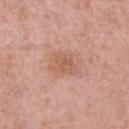  biopsy_status: not biopsied; imaged during a skin examination